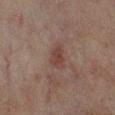This lesion was catalogued during total-body skin photography and was not selected for biopsy.
Measured at roughly 2.5 mm in maximum diameter.
The lesion is on the leg.
A female patient, aged 78–82.
A close-up tile cropped from a whole-body skin photograph, about 15 mm across.